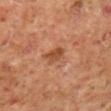Recorded during total-body skin imaging; not selected for excision or biopsy.
Captured under cross-polarized illumination.
A male patient aged around 60.
Approximately 2.5 mm at its widest.
The lesion is located on the leg.
A 15 mm close-up extracted from a 3D total-body photography capture.